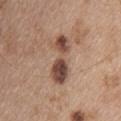The lesion was tiled from a total-body skin photograph and was not biopsied. The subject is a female about 45 years old. Approximately 6 mm at its widest. On the upper back. Captured under white-light illumination. A 15 mm crop from a total-body photograph taken for skin-cancer surveillance. Automated image analysis of the tile measured a mean CIELAB color near L≈47 a*≈19 b*≈27 and a normalized lesion–skin contrast near 10. And it measured an automated nevus-likeness rating near 85 out of 100 and a detector confidence of about 100 out of 100 that the crop contains a lesion.